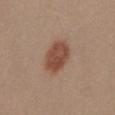Q: Was this lesion biopsied?
A: imaged on a skin check; not biopsied
Q: What lighting was used for the tile?
A: white-light
Q: Who is the patient?
A: female, aged approximately 30
Q: How was this image acquired?
A: total-body-photography crop, ~15 mm field of view
Q: Where on the body is the lesion?
A: the mid back
Q: What did automated image analysis measure?
A: a shape eccentricity near 0.7; a mean CIELAB color near L≈48 a*≈21 b*≈28 and a normalized lesion–skin contrast near 8.5; a border-irregularity rating of about 1.5/10, a color-variation rating of about 3.5/10, and peripheral color asymmetry of about 1; a classifier nevus-likeness of about 100/100